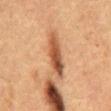follow-up = total-body-photography surveillance lesion; no biopsy | image = total-body-photography crop, ~15 mm field of view | subject = male, in their mid-60s | tile lighting = cross-polarized illumination | body site = the mid back | image-analysis metrics = a footprint of about 10 mm² and a symmetry-axis asymmetry near 0.15; border irregularity of about 3 on a 0–10 scale, a color-variation rating of about 6/10, and a peripheral color-asymmetry measure near 2; a classifier nevus-likeness of about 100/100 and a lesion-detection confidence of about 80/100 | lesion size = ~6 mm (longest diameter).